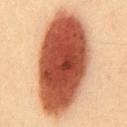notes: imaged on a skin check; not biopsied
body site: the front of the torso
patient: male, aged 33 to 37
imaging modality: total-body-photography crop, ~15 mm field of view
automated lesion analysis: a lesion color around L≈47 a*≈27 b*≈32 in CIELAB and roughly 23 lightness units darker than nearby skin; a border-irregularity index near 1.5/10, a color-variation rating of about 7.5/10, and radial color variation of about 2; a classifier nevus-likeness of about 100/100 and a detector confidence of about 100 out of 100 that the crop contains a lesion